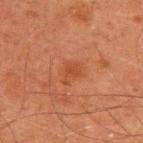Assessment: Part of a total-body skin-imaging series; this lesion was reviewed on a skin check and was not flagged for biopsy. Image and clinical context: The subject is a male aged 43–47. An algorithmic analysis of the crop reported a lesion area of about 4.5 mm², a shape eccentricity near 0.7, and two-axis asymmetry of about 0.4. The analysis additionally found about 5 CIELAB-L* units darker than the surrounding skin and a normalized border contrast of about 5.5. The software also gave a nevus-likeness score of about 5/100. The lesion is located on the upper back. Imaged with cross-polarized lighting. A close-up tile cropped from a whole-body skin photograph, about 15 mm across. Approximately 2.5 mm at its widest.